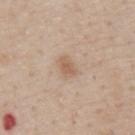Assessment: Part of a total-body skin-imaging series; this lesion was reviewed on a skin check and was not flagged for biopsy. Clinical summary: The lesion is on the abdomen. A region of skin cropped from a whole-body photographic capture, roughly 15 mm wide. About 2.5 mm across. A male subject in their 60s. This is a white-light tile.